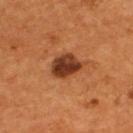Part of a total-body skin-imaging series; this lesion was reviewed on a skin check and was not flagged for biopsy.
The lesion's longest dimension is about 4 mm.
Cropped from a total-body skin-imaging series; the visible field is about 15 mm.
The lesion is on the back.
The patient is a male in their mid- to late 50s.